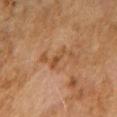Q: Was this lesion biopsied?
A: catalogued during a skin exam; not biopsied
Q: How was this image acquired?
A: 15 mm crop, total-body photography
Q: Who is the patient?
A: female, aged around 60
Q: What is the anatomic site?
A: the right upper arm